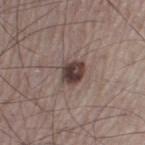biopsy_status: not biopsied; imaged during a skin examination
image:
  source: total-body photography crop
  field_of_view_mm: 15
lesion_size:
  long_diameter_mm_approx: 3.0
patient:
  sex: male
  age_approx: 75
lighting: white-light
site: leg
automated_metrics:
  vs_skin_darker_L: 15.0
  vs_skin_contrast_norm: 11.5
  border_irregularity_0_10: 2.0
  color_variation_0_10: 5.0
  peripheral_color_asymmetry: 2.0
  nevus_likeness_0_100: 85
  lesion_detection_confidence_0_100: 100